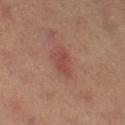Q: Was this lesion biopsied?
A: no biopsy performed (imaged during a skin exam)
Q: Who is the patient?
A: female, roughly 40 years of age
Q: How was this image acquired?
A: ~15 mm crop, total-body skin-cancer survey
Q: Where on the body is the lesion?
A: the right lower leg